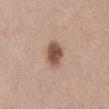Imaged during a routine full-body skin examination; the lesion was not biopsied and no histopathology is available. A 15 mm close-up tile from a total-body photography series done for melanoma screening. The subject is a male roughly 50 years of age. The lesion-visualizer software estimated a lesion area of about 7 mm², an eccentricity of roughly 0.75, and a symmetry-axis asymmetry near 0.2. The analysis additionally found roughly 15 lightness units darker than nearby skin and a normalized lesion–skin contrast near 10.5. It also reported an automated nevus-likeness rating near 100 out of 100 and a detector confidence of about 100 out of 100 that the crop contains a lesion. From the abdomen.